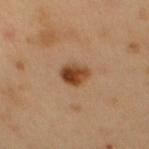notes: imaged on a skin check; not biopsied | lesion size: about 3 mm | anatomic site: the chest | automated metrics: an area of roughly 6.5 mm², an outline eccentricity of about 0.5 (0 = round, 1 = elongated), and two-axis asymmetry of about 0.15; a mean CIELAB color near L≈44 a*≈21 b*≈35, roughly 14 lightness units darker than nearby skin, and a normalized border contrast of about 10.5; a border-irregularity rating of about 1.5/10 and a color-variation rating of about 7.5/10 | subject: male, approximately 50 years of age | imaging modality: 15 mm crop, total-body photography.